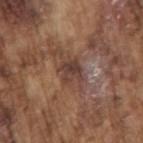{"biopsy_status": "not biopsied; imaged during a skin examination", "image": {"source": "total-body photography crop", "field_of_view_mm": 15}, "site": "right upper arm", "patient": {"sex": "male", "age_approx": 75}, "lighting": "white-light", "lesion_size": {"long_diameter_mm_approx": 3.0}}